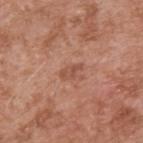Assessment: This lesion was catalogued during total-body skin photography and was not selected for biopsy. Image and clinical context: A 15 mm close-up extracted from a 3D total-body photography capture. The lesion is located on the upper back. A male patient roughly 55 years of age.